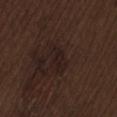notes: no biopsy performed (imaged during a skin exam)
image: 15 mm crop, total-body photography
illumination: white-light illumination
lesion size: ≈2.5 mm
body site: the lower back
TBP lesion metrics: a footprint of about 2.5 mm², a shape eccentricity near 0.9, and two-axis asymmetry of about 0.25; a lesion color around L≈17 a*≈14 b*≈15 in CIELAB and roughly 4 lightness units darker than nearby skin; a border-irregularity rating of about 2.5/10, a within-lesion color-variation index near 1.5/10, and peripheral color asymmetry of about 0.5
patient: male, approximately 70 years of age